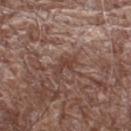Q: Was this lesion biopsied?
A: imaged on a skin check; not biopsied
Q: Patient demographics?
A: male, roughly 70 years of age
Q: How was this image acquired?
A: ~15 mm crop, total-body skin-cancer survey
Q: What is the anatomic site?
A: the left thigh
Q: What lighting was used for the tile?
A: white-light illumination
Q: What did automated image analysis measure?
A: a footprint of about 3.5 mm², an outline eccentricity of about 0.85 (0 = round, 1 = elongated), and two-axis asymmetry of about 0.45; a lesion color around L≈41 a*≈20 b*≈24 in CIELAB, roughly 8 lightness units darker than nearby skin, and a normalized border contrast of about 6.5
Q: How large is the lesion?
A: about 3 mm